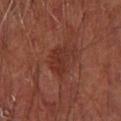Clinical summary:
The lesion is on the leg. The patient is a male roughly 65 years of age. Approximately 4 mm at its widest. Cropped from a whole-body photographic skin survey; the tile spans about 15 mm. An algorithmic analysis of the crop reported an outline eccentricity of about 0.75 (0 = round, 1 = elongated) and two-axis asymmetry of about 0.3. It also reported an average lesion color of about L≈30 a*≈23 b*≈26 (CIELAB) and a lesion-to-skin contrast of about 6 (normalized; higher = more distinct). The software also gave a border-irregularity index near 3.5/10, a color-variation rating of about 2.5/10, and peripheral color asymmetry of about 1. The software also gave a nevus-likeness score of about 0/100.